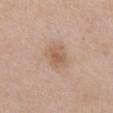<record>
  <biopsy_status>not biopsied; imaged during a skin examination</biopsy_status>
  <patient>
    <sex>female</sex>
    <age_approx>60</age_approx>
  </patient>
  <image>
    <source>total-body photography crop</source>
    <field_of_view_mm>15</field_of_view_mm>
  </image>
  <lighting>white-light</lighting>
  <lesion_size>
    <long_diameter_mm_approx>3.5</long_diameter_mm_approx>
  </lesion_size>
  <site>mid back</site>
</record>